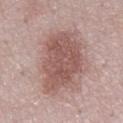No biopsy was performed on this lesion — it was imaged during a full skin examination and was not determined to be concerning. Automated image analysis of the tile measured a footprint of about 29 mm², an eccentricity of roughly 0.8, and a symmetry-axis asymmetry near 0.25. And it measured roughly 12 lightness units darker than nearby skin and a normalized border contrast of about 8.5. And it measured a border-irregularity index near 3.5/10, a within-lesion color-variation index near 3/10, and a peripheral color-asymmetry measure near 1. Longest diameter approximately 8 mm. On the mid back. A 15 mm close-up extracted from a 3D total-body photography capture. A male patient, about 55 years old. This is a white-light tile.